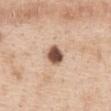Assessment: Part of a total-body skin-imaging series; this lesion was reviewed on a skin check and was not flagged for biopsy. Background: A female subject aged 38 to 42. From the front of the torso. Automated tile analysis of the lesion measured a footprint of about 5.5 mm² and an eccentricity of roughly 0.45. Cropped from a whole-body photographic skin survey; the tile spans about 15 mm.